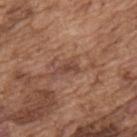Part of a total-body skin-imaging series; this lesion was reviewed on a skin check and was not flagged for biopsy.
This is a white-light tile.
The lesion is located on the upper back.
Cropped from a whole-body photographic skin survey; the tile spans about 15 mm.
A male subject, aged approximately 75.
Automated tile analysis of the lesion measured an eccentricity of roughly 0.9 and two-axis asymmetry of about 0.5. It also reported internal color variation of about 0 on a 0–10 scale and a peripheral color-asymmetry measure near 0.